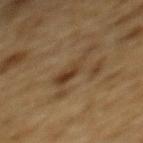Q: Was this lesion biopsied?
A: no biopsy performed (imaged during a skin exam)
Q: What is the anatomic site?
A: the back
Q: What is the imaging modality?
A: total-body-photography crop, ~15 mm field of view
Q: Patient demographics?
A: male, in their mid-80s or older